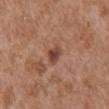Impression:
No biopsy was performed on this lesion — it was imaged during a full skin examination and was not determined to be concerning.
Background:
A lesion tile, about 15 mm wide, cut from a 3D total-body photograph. Imaged with white-light lighting. A male subject, aged around 75. On the abdomen.